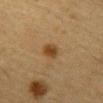{"biopsy_status": "not biopsied; imaged during a skin examination", "site": "abdomen", "patient": {"sex": "male", "age_approx": "85+"}, "lesion_size": {"long_diameter_mm_approx": 2.5}, "lighting": "cross-polarized", "image": {"source": "total-body photography crop", "field_of_view_mm": 15}}